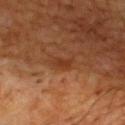biopsy status = total-body-photography surveillance lesion; no biopsy.